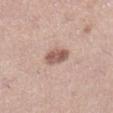No biopsy was performed on this lesion — it was imaged during a full skin examination and was not determined to be concerning. An algorithmic analysis of the crop reported a border-irregularity index near 1.5/10, a color-variation rating of about 3.5/10, and a peripheral color-asymmetry measure near 1. And it measured a classifier nevus-likeness of about 85/100 and a lesion-detection confidence of about 100/100. A 15 mm close-up tile from a total-body photography series done for melanoma screening. About 3 mm across. Located on the right lower leg. Captured under white-light illumination. The patient is a female aged 33–37.